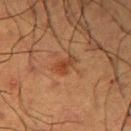Captured during whole-body skin photography for melanoma surveillance; the lesion was not biopsied.
About 3 mm across.
A lesion tile, about 15 mm wide, cut from a 3D total-body photograph.
A male patient about 60 years old.
The tile uses cross-polarized illumination.
The lesion is located on the right upper arm.
An algorithmic analysis of the crop reported internal color variation of about 1.5 on a 0–10 scale and a peripheral color-asymmetry measure near 0.5. It also reported a classifier nevus-likeness of about 5/100 and a lesion-detection confidence of about 100/100.